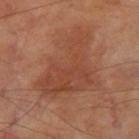Findings:
* workup · imaged on a skin check; not biopsied
* site · the leg
* illumination · cross-polarized illumination
* automated metrics · a border-irregularity index near 6/10, a within-lesion color-variation index near 3.5/10, and a peripheral color-asymmetry measure near 1; a classifier nevus-likeness of about 0/100 and a detector confidence of about 100 out of 100 that the crop contains a lesion
* diameter · about 9 mm
* subject · male, roughly 70 years of age
* imaging modality · 15 mm crop, total-body photography